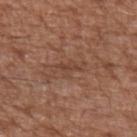biopsy status: imaged on a skin check; not biopsied | site: the left upper arm | patient: male, aged 73 to 77 | lesion diameter: ~3 mm (longest diameter) | lighting: white-light illumination | acquisition: total-body-photography crop, ~15 mm field of view.